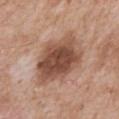{
  "biopsy_status": "not biopsied; imaged during a skin examination",
  "patient": {
    "sex": "male",
    "age_approx": 60
  },
  "image": {
    "source": "total-body photography crop",
    "field_of_view_mm": 15
  },
  "site": "chest"
}